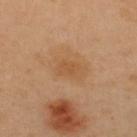{
  "biopsy_status": "not biopsied; imaged during a skin examination",
  "image": {
    "source": "total-body photography crop",
    "field_of_view_mm": 15
  },
  "site": "back",
  "lighting": "cross-polarized",
  "automated_metrics": {
    "lesion_detection_confidence_0_100": 100
  },
  "lesion_size": {
    "long_diameter_mm_approx": 2.5
  },
  "patient": {
    "sex": "female",
    "age_approx": 40
  }
}